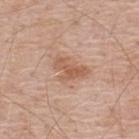The lesion was photographed on a routine skin check and not biopsied; there is no pathology result. A roughly 15 mm field-of-view crop from a total-body skin photograph. From the back. The tile uses white-light illumination. Approximately 4 mm at its widest. A male subject in their 50s.